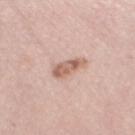The lesion was tiled from a total-body skin photograph and was not biopsied. The subject is a female approximately 65 years of age. A region of skin cropped from a whole-body photographic capture, roughly 15 mm wide. The tile uses white-light illumination. On the left thigh. Automated image analysis of the tile measured a normalized lesion–skin contrast near 8.